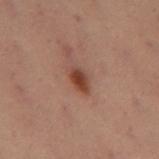The lesion was tiled from a total-body skin photograph and was not biopsied. A female patient, in their mid-50s. The lesion is located on the left thigh. A close-up tile cropped from a whole-body skin photograph, about 15 mm across.